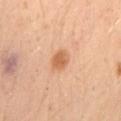Impression: No biopsy was performed on this lesion — it was imaged during a full skin examination and was not determined to be concerning. Background: A 15 mm crop from a total-body photograph taken for skin-cancer surveillance. The lesion is on the lower back. A male patient about 30 years old. Captured under cross-polarized illumination.